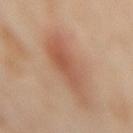<tbp_lesion>
<biopsy_status>not biopsied; imaged during a skin examination</biopsy_status>
<lesion_size>
  <long_diameter_mm_approx>7.0</long_diameter_mm_approx>
</lesion_size>
<image>
  <source>total-body photography crop</source>
  <field_of_view_mm>15</field_of_view_mm>
</image>
<lighting>cross-polarized</lighting>
<automated_metrics>
  <color_variation_0_10>5.0</color_variation_0_10>
  <peripheral_color_asymmetry>1.5</peripheral_color_asymmetry>
</automated_metrics>
<patient>
  <sex>female</sex>
  <age_approx>55</age_approx>
</patient>
<site>back</site>
</tbp_lesion>